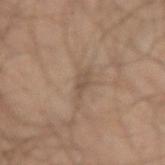Captured during whole-body skin photography for melanoma surveillance; the lesion was not biopsied. The tile uses cross-polarized illumination. A 15 mm close-up tile from a total-body photography series done for melanoma screening. The lesion-visualizer software estimated a lesion area of about 2.5 mm², an eccentricity of roughly 0.85, and a shape-asymmetry score of about 0.35 (0 = symmetric). And it measured a mean CIELAB color near L≈47 a*≈13 b*≈26, a lesion–skin lightness drop of about 7, and a normalized border contrast of about 5.5. And it measured a border-irregularity index near 4/10 and radial color variation of about 0. A male subject, aged 33–37. The lesion is located on the left arm. About 2.5 mm across.